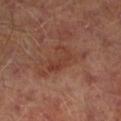The lesion was tiled from a total-body skin photograph and was not biopsied. The tile uses cross-polarized illumination. A male subject, roughly 65 years of age. The lesion's longest dimension is about 4 mm. A roughly 15 mm field-of-view crop from a total-body skin photograph. On the left lower leg.